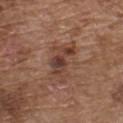Q: Is there a histopathology result?
A: no biopsy performed (imaged during a skin exam)
Q: What did automated image analysis measure?
A: a lesion area of about 11 mm², a shape eccentricity near 0.85, and a symmetry-axis asymmetry near 0.45; a nevus-likeness score of about 30/100
Q: What kind of image is this?
A: total-body-photography crop, ~15 mm field of view
Q: Who is the patient?
A: male, about 75 years old
Q: What lighting was used for the tile?
A: white-light
Q: What is the anatomic site?
A: the chest
Q: Lesion size?
A: ≈5.5 mm